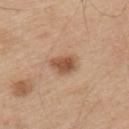The lesion was tiled from a total-body skin photograph and was not biopsied. The recorded lesion diameter is about 3.5 mm. Imaged with white-light lighting. Located on the upper back. A 15 mm crop from a total-body photograph taken for skin-cancer surveillance. A male subject aged 53–57. An algorithmic analysis of the crop reported a lesion color around L≈53 a*≈20 b*≈33 in CIELAB, about 12 CIELAB-L* units darker than the surrounding skin, and a normalized border contrast of about 8.5. The analysis additionally found a border-irregularity rating of about 2.5/10 and a within-lesion color-variation index near 3/10.